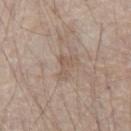{"biopsy_status": "not biopsied; imaged during a skin examination", "image": {"source": "total-body photography crop", "field_of_view_mm": 15}, "site": "chest", "patient": {"sex": "male", "age_approx": 80}}